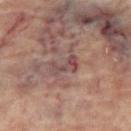Case summary:
- biopsy status · imaged on a skin check; not biopsied
- tile lighting · cross-polarized
- location · the left leg
- image source · ~15 mm tile from a whole-body skin photo
- TBP lesion metrics · an area of roughly 5 mm², an outline eccentricity of about 0.85 (0 = round, 1 = elongated), and a shape-asymmetry score of about 0.4 (0 = symmetric); a border-irregularity index near 5/10, internal color variation of about 3 on a 0–10 scale, and radial color variation of about 1
- subject · female, aged 63–67